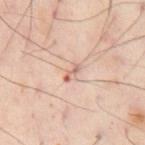Part of a total-body skin-imaging series; this lesion was reviewed on a skin check and was not flagged for biopsy. A male subject roughly 55 years of age. Imaged with cross-polarized lighting. Measured at roughly 2.5 mm in maximum diameter. From the front of the torso. The total-body-photography lesion software estimated a footprint of about 2 mm², a shape eccentricity near 0.95, and two-axis asymmetry of about 0.4. The analysis additionally found a lesion color around L≈65 a*≈23 b*≈28 in CIELAB, about 11 CIELAB-L* units darker than the surrounding skin, and a normalized border contrast of about 6.5. The software also gave border irregularity of about 4.5 on a 0–10 scale, internal color variation of about 0 on a 0–10 scale, and radial color variation of about 0. A 15 mm close-up extracted from a 3D total-body photography capture.